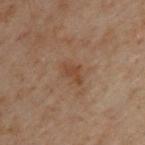<tbp_lesion>
<biopsy_status>not biopsied; imaged during a skin examination</biopsy_status>
<lesion_size>
  <long_diameter_mm_approx>3.0</long_diameter_mm_approx>
</lesion_size>
<site>upper back</site>
<lighting>cross-polarized</lighting>
<patient>
  <sex>male</sex>
  <age_approx>50</age_approx>
</patient>
<image>
  <source>total-body photography crop</source>
  <field_of_view_mm>15</field_of_view_mm>
</image>
</tbp_lesion>